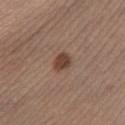<case>
  <biopsy_status>not biopsied; imaged during a skin examination</biopsy_status>
  <lighting>white-light</lighting>
  <lesion_size>
    <long_diameter_mm_approx>2.5</long_diameter_mm_approx>
  </lesion_size>
  <patient>
    <sex>male</sex>
    <age_approx>35</age_approx>
  </patient>
  <site>left lower leg</site>
  <image>
    <source>total-body photography crop</source>
    <field_of_view_mm>15</field_of_view_mm>
  </image>
</case>